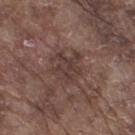Q: Was this lesion biopsied?
A: imaged on a skin check; not biopsied
Q: What kind of image is this?
A: 15 mm crop, total-body photography
Q: What did automated image analysis measure?
A: a shape eccentricity near 0.45 and a shape-asymmetry score of about 0.45 (0 = symmetric); a nevus-likeness score of about 0/100 and a lesion-detection confidence of about 95/100
Q: Who is the patient?
A: male, aged approximately 75
Q: What lighting was used for the tile?
A: white-light illumination
Q: What is the anatomic site?
A: the right thigh